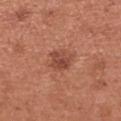| key | value |
|---|---|
| follow-up | no biopsy performed (imaged during a skin exam) |
| image source | 15 mm crop, total-body photography |
| tile lighting | white-light |
| patient | female, aged around 50 |
| location | the arm |